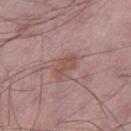Clinical impression: Part of a total-body skin-imaging series; this lesion was reviewed on a skin check and was not flagged for biopsy. Acquisition and patient details: A region of skin cropped from a whole-body photographic capture, roughly 15 mm wide. The lesion is on the right thigh. A male patient aged 48 to 52.